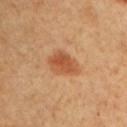• workup: catalogued during a skin exam; not biopsied
• lesion diameter: about 3.5 mm
• image-analysis metrics: a mean CIELAB color near L≈53 a*≈26 b*≈38, a lesion–skin lightness drop of about 11, and a normalized border contrast of about 7.5; border irregularity of about 2 on a 0–10 scale, a color-variation rating of about 3.5/10, and radial color variation of about 1; an automated nevus-likeness rating near 100 out of 100 and a detector confidence of about 100 out of 100 that the crop contains a lesion
• subject: male, aged approximately 50
• lighting: cross-polarized
• body site: the left upper arm
• image source: 15 mm crop, total-body photography